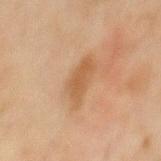The lesion was tiled from a total-body skin photograph and was not biopsied. A 15 mm close-up tile from a total-body photography series done for melanoma screening. Located on the mid back. The lesion's longest dimension is about 5 mm. A male subject in their mid- to late 40s. Captured under cross-polarized illumination.